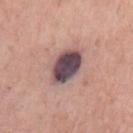Captured during whole-body skin photography for melanoma surveillance; the lesion was not biopsied. Located on the arm. The recorded lesion diameter is about 4.5 mm. The subject is a female aged 63 to 67. Cropped from a total-body skin-imaging series; the visible field is about 15 mm.